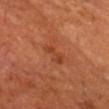No biopsy was performed on this lesion — it was imaged during a full skin examination and was not determined to be concerning.
Measured at roughly 3.5 mm in maximum diameter.
Cropped from a total-body skin-imaging series; the visible field is about 15 mm.
The total-body-photography lesion software estimated a border-irregularity rating of about 4.5/10 and radial color variation of about 0.5. And it measured a lesion-detection confidence of about 100/100.
Imaged with cross-polarized lighting.
A male subject in their mid-60s.
Located on the head or neck.